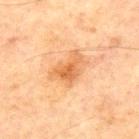Part of a total-body skin-imaging series; this lesion was reviewed on a skin check and was not flagged for biopsy. A male patient, aged approximately 65. An algorithmic analysis of the crop reported an average lesion color of about L≈52 a*≈22 b*≈37 (CIELAB), roughly 9 lightness units darker than nearby skin, and a normalized border contrast of about 7. The software also gave a border-irregularity rating of about 5.5/10, a color-variation rating of about 3.5/10, and a peripheral color-asymmetry measure near 1. Cropped from a whole-body photographic skin survey; the tile spans about 15 mm. From the chest. Approximately 3.5 mm at its widest.